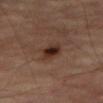{
  "biopsy_status": "not biopsied; imaged during a skin examination",
  "patient": {
    "sex": "male",
    "age_approx": 70
  },
  "image": {
    "source": "total-body photography crop",
    "field_of_view_mm": 15
  },
  "site": "right thigh",
  "lighting": "cross-polarized"
}